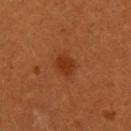body site = the upper back | imaging modality = ~15 mm tile from a whole-body skin photo | subject = female, aged 28–32.